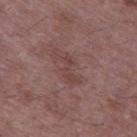Findings:
• workup: no biopsy performed (imaged during a skin exam)
• automated lesion analysis: an area of roughly 5 mm² and a shape eccentricity near 0.9
• image source: 15 mm crop, total-body photography
• patient: male, aged 48–52
• location: the left thigh